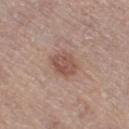biopsy status = imaged on a skin check; not biopsied | location = the leg | imaging modality = total-body-photography crop, ~15 mm field of view | diameter = ~3 mm (longest diameter) | subject = female, aged 63 to 67 | illumination = white-light.